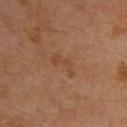image source: total-body-photography crop, ~15 mm field of view
patient: male, about 70 years old
diameter: about 3.5 mm
automated lesion analysis: an area of roughly 3.5 mm², an outline eccentricity of about 0.9 (0 = round, 1 = elongated), and a shape-asymmetry score of about 0.55 (0 = symmetric); a mean CIELAB color near L≈43 a*≈20 b*≈33 and a normalized lesion–skin contrast near 5; a classifier nevus-likeness of about 0/100
site: the upper back